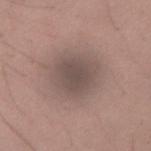Clinical impression: Recorded during total-body skin imaging; not selected for excision or biopsy. Background: A roughly 15 mm field-of-view crop from a total-body skin photograph. Measured at roughly 4.5 mm in maximum diameter. A male patient approximately 35 years of age. Captured under white-light illumination. On the arm.